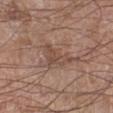notes: catalogued during a skin exam; not biopsied | location: the right lower leg | subject: male, aged approximately 60 | image: ~15 mm tile from a whole-body skin photo.